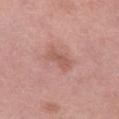<lesion>
  <biopsy_status>not biopsied; imaged during a skin examination</biopsy_status>
  <lesion_size>
    <long_diameter_mm_approx>3.5</long_diameter_mm_approx>
  </lesion_size>
  <automated_metrics>
    <shape_asymmetry>0.35</shape_asymmetry>
    <color_variation_0_10>0.5</color_variation_0_10>
    <peripheral_color_asymmetry>0.0</peripheral_color_asymmetry>
    <nevus_likeness_0_100>0</nevus_likeness_0_100>
    <lesion_detection_confidence_0_100>100</lesion_detection_confidence_0_100>
  </automated_metrics>
  <patient>
    <sex>female</sex>
    <age_approx>50</age_approx>
  </patient>
  <image>
    <source>total-body photography crop</source>
    <field_of_view_mm>15</field_of_view_mm>
  </image>
  <lighting>white-light</lighting>
  <site>left thigh</site>
</lesion>